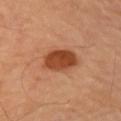The lesion was tiled from a total-body skin photograph and was not biopsied. The recorded lesion diameter is about 4 mm. This is a cross-polarized tile. A 15 mm close-up extracted from a 3D total-body photography capture. Located on the right upper arm.